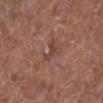  biopsy_status: not biopsied; imaged during a skin examination
  image:
    source: total-body photography crop
    field_of_view_mm: 15
  site: left lower leg
  lighting: white-light
  patient:
    sex: female
    age_approx: 80